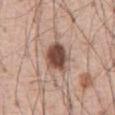Q: Is there a histopathology result?
A: imaged on a skin check; not biopsied
Q: Patient demographics?
A: male, aged 53 to 57
Q: How was the tile lit?
A: white-light
Q: Lesion size?
A: ~4 mm (longest diameter)
Q: What is the anatomic site?
A: the abdomen
Q: How was this image acquired?
A: 15 mm crop, total-body photography